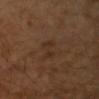Impression: Recorded during total-body skin imaging; not selected for excision or biopsy. Image and clinical context: A region of skin cropped from a whole-body photographic capture, roughly 15 mm wide. A male patient about 65 years old.